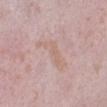{
  "biopsy_status": "not biopsied; imaged during a skin examination",
  "lesion_size": {
    "long_diameter_mm_approx": 5.5
  },
  "image": {
    "source": "total-body photography crop",
    "field_of_view_mm": 15
  },
  "lighting": "white-light",
  "patient": {
    "sex": "female",
    "age_approx": 40
  },
  "site": "leg"
}